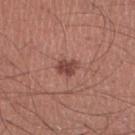This lesion was catalogued during total-body skin photography and was not selected for biopsy. This image is a 15 mm lesion crop taken from a total-body photograph. This is a white-light tile. A male subject aged around 45. The lesion's longest dimension is about 2.5 mm. The lesion is located on the left lower leg.